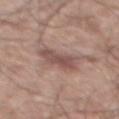The lesion was tiled from a total-body skin photograph and was not biopsied. A region of skin cropped from a whole-body photographic capture, roughly 15 mm wide. A male subject, aged 53–57. On the mid back.